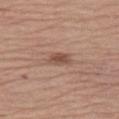Notes:
– notes: no biopsy performed (imaged during a skin exam)
– patient: female, in their mid-60s
– TBP lesion metrics: a classifier nevus-likeness of about 80/100
– site: the left thigh
– image source: ~15 mm crop, total-body skin-cancer survey